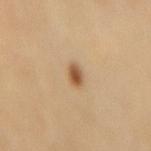Clinical impression:
No biopsy was performed on this lesion — it was imaged during a full skin examination and was not determined to be concerning.
Clinical summary:
From the mid back. Approximately 2.5 mm at its widest. Cropped from a whole-body photographic skin survey; the tile spans about 15 mm. A female subject, aged around 70.